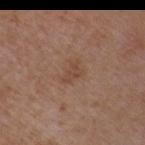A male patient aged around 50.
The tile uses white-light illumination.
Cropped from a whole-body photographic skin survey; the tile spans about 15 mm.
On the left upper arm.